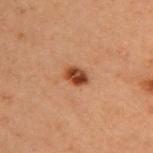{
  "biopsy_status": "not biopsied; imaged during a skin examination",
  "lesion_size": {
    "long_diameter_mm_approx": 2.5
  },
  "site": "back",
  "automated_metrics": {
    "area_mm2_approx": 4.0,
    "eccentricity": 0.7,
    "shape_asymmetry": 0.2,
    "cielab_L": 36,
    "cielab_a": 23,
    "cielab_b": 31,
    "vs_skin_darker_L": 14.0,
    "border_irregularity_0_10": 2.0,
    "color_variation_0_10": 4.5,
    "nevus_likeness_0_100": 100,
    "lesion_detection_confidence_0_100": 100
  },
  "patient": {
    "sex": "female",
    "age_approx": 40
  },
  "image": {
    "source": "total-body photography crop",
    "field_of_view_mm": 15
  }
}